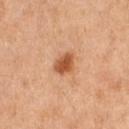– follow-up — total-body-photography surveillance lesion; no biopsy
– acquisition — total-body-photography crop, ~15 mm field of view
– subject — female, about 60 years old
– illumination — cross-polarized
– body site — the right thigh
– automated metrics — border irregularity of about 2.5 on a 0–10 scale, a color-variation rating of about 2.5/10, and a peripheral color-asymmetry measure near 0.5; a lesion-detection confidence of about 100/100
– lesion size — ≈3 mm